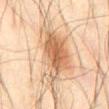notes: catalogued during a skin exam; not biopsied
size: about 11.5 mm
patient: male, aged approximately 45
acquisition: ~15 mm crop, total-body skin-cancer survey
location: the abdomen
lighting: cross-polarized